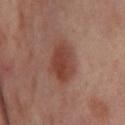notes — catalogued during a skin exam; not biopsied
imaging modality — total-body-photography crop, ~15 mm field of view
illumination — cross-polarized
location — the left thigh
automated metrics — a footprint of about 12 mm², a shape eccentricity near 0.7, and a shape-asymmetry score of about 0.15 (0 = symmetric); an average lesion color of about L≈43 a*≈24 b*≈27 (CIELAB), about 10 CIELAB-L* units darker than the surrounding skin, and a lesion-to-skin contrast of about 8 (normalized; higher = more distinct)
diameter — about 4.5 mm
subject — female, about 55 years old